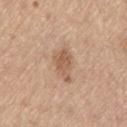The lesion was tiled from a total-body skin photograph and was not biopsied.